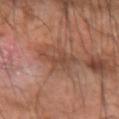Q: Was this lesion biopsied?
A: total-body-photography surveillance lesion; no biopsy
Q: Illumination type?
A: cross-polarized
Q: How was this image acquired?
A: total-body-photography crop, ~15 mm field of view
Q: Lesion size?
A: about 4 mm
Q: Lesion location?
A: the left forearm
Q: What are the patient's age and sex?
A: male, aged 58–62